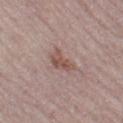Clinical impression: The lesion was photographed on a routine skin check and not biopsied; there is no pathology result. Context: The total-body-photography lesion software estimated a footprint of about 4.5 mm² and a shape-asymmetry score of about 0.45 (0 = symmetric). And it measured roughly 10 lightness units darker than nearby skin and a lesion-to-skin contrast of about 7.5 (normalized; higher = more distinct). It also reported border irregularity of about 5 on a 0–10 scale, a within-lesion color-variation index near 2.5/10, and radial color variation of about 1. Located on the left thigh. The tile uses white-light illumination. The lesion's longest dimension is about 3.5 mm. The subject is a female aged 63–67. A close-up tile cropped from a whole-body skin photograph, about 15 mm across.